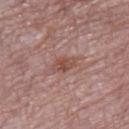notes — catalogued during a skin exam; not biopsied | location — the leg | subject — female, aged 68 to 72 | image source — 15 mm crop, total-body photography | lighting — white-light.